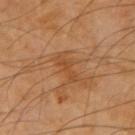workup: total-body-photography surveillance lesion; no biopsy | anatomic site: the left forearm | patient: male, roughly 70 years of age | lesion size: about 4 mm | imaging modality: ~15 mm crop, total-body skin-cancer survey | image-analysis metrics: a lesion area of about 5.5 mm² and a symmetry-axis asymmetry near 0.35; a lesion color around L≈45 a*≈22 b*≈37 in CIELAB, about 6 CIELAB-L* units darker than the surrounding skin, and a normalized border contrast of about 5.5; a border-irregularity index near 5/10 and a within-lesion color-variation index near 1/10; an automated nevus-likeness rating near 0 out of 100 and a detector confidence of about 100 out of 100 that the crop contains a lesion | lighting: cross-polarized illumination.